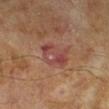Captured during whole-body skin photography for melanoma surveillance; the lesion was not biopsied. A close-up tile cropped from a whole-body skin photograph, about 15 mm across. Longest diameter approximately 4.5 mm. The total-body-photography lesion software estimated roughly 7 lightness units darker than nearby skin and a normalized border contrast of about 7. The software also gave border irregularity of about 3 on a 0–10 scale, internal color variation of about 7 on a 0–10 scale, and a peripheral color-asymmetry measure near 2. The subject is a male about 65 years old. The tile uses cross-polarized illumination.